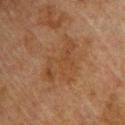Findings:
- acquisition: total-body-photography crop, ~15 mm field of view
- body site: the upper back
- TBP lesion metrics: a lesion area of about 12 mm² and two-axis asymmetry of about 0.6; a lesion color around L≈37 a*≈18 b*≈29 in CIELAB and about 5 CIELAB-L* units darker than the surrounding skin; a border-irregularity rating of about 9.5/10, internal color variation of about 2.5 on a 0–10 scale, and radial color variation of about 0.5; an automated nevus-likeness rating near 0 out of 100
- patient: male, in their mid-70s